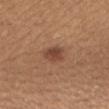No biopsy was performed on this lesion — it was imaged during a full skin examination and was not determined to be concerning. Cropped from a whole-body photographic skin survey; the tile spans about 15 mm. A female patient aged 33 to 37. From the mid back.